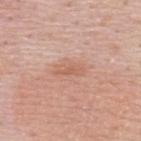Image and clinical context: An algorithmic analysis of the crop reported an area of roughly 4 mm² and a shape eccentricity near 0.9. The analysis additionally found an average lesion color of about L≈60 a*≈23 b*≈31 (CIELAB), a lesion–skin lightness drop of about 7, and a normalized lesion–skin contrast near 5.5. And it measured border irregularity of about 4 on a 0–10 scale, a color-variation rating of about 1/10, and radial color variation of about 0. It also reported an automated nevus-likeness rating near 0 out of 100 and lesion-presence confidence of about 100/100. Captured under white-light illumination. The lesion is located on the upper back. A male patient, in their 50s. A 15 mm close-up tile from a total-body photography series done for melanoma screening.